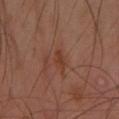Impression:
Imaged during a routine full-body skin examination; the lesion was not biopsied and no histopathology is available.
Image and clinical context:
The lesion is on the left forearm. This is a cross-polarized tile. The patient is a female approximately 55 years of age. A close-up tile cropped from a whole-body skin photograph, about 15 mm across. About 2.5 mm across.